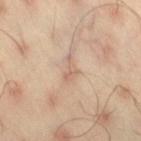{
  "automated_metrics": {
    "cielab_L": 62,
    "cielab_a": 18,
    "cielab_b": 28,
    "vs_skin_darker_L": 8.0,
    "vs_skin_contrast_norm": 5.0,
    "border_irregularity_0_10": 7.0,
    "color_variation_0_10": 0.0
  },
  "site": "right thigh",
  "patient": {
    "sex": "male",
    "age_approx": 45
  },
  "image": {
    "source": "total-body photography crop",
    "field_of_view_mm": 15
  }
}